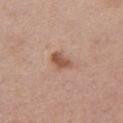notes = total-body-photography surveillance lesion; no biopsy | size = ≈3 mm | subject = female, in their 40s | TBP lesion metrics = a mean CIELAB color near L≈53 a*≈21 b*≈30; an automated nevus-likeness rating near 65 out of 100 and a detector confidence of about 100 out of 100 that the crop contains a lesion | body site = the chest | image source = ~15 mm crop, total-body skin-cancer survey.